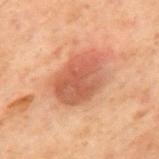This lesion was catalogued during total-body skin photography and was not selected for biopsy. A 15 mm close-up extracted from a 3D total-body photography capture. An algorithmic analysis of the crop reported an outline eccentricity of about 0.75 (0 = round, 1 = elongated) and a symmetry-axis asymmetry near 0.15. The software also gave a nevus-likeness score of about 65/100 and a lesion-detection confidence of about 100/100. The tile uses cross-polarized illumination. A male subject aged 68 to 72. Longest diameter approximately 6 mm. The lesion is located on the mid back.